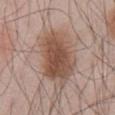No biopsy was performed on this lesion — it was imaged during a full skin examination and was not determined to be concerning. The patient is a male aged around 55. This is a white-light tile. A roughly 15 mm field-of-view crop from a total-body skin photograph. On the abdomen. The lesion's longest dimension is about 7.5 mm.